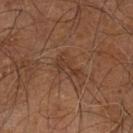Part of a total-body skin-imaging series; this lesion was reviewed on a skin check and was not flagged for biopsy.
From the right leg.
Approximately 3.5 mm at its widest.
This image is a 15 mm lesion crop taken from a total-body photograph.
The patient is a male aged around 60.
The total-body-photography lesion software estimated border irregularity of about 4.5 on a 0–10 scale, a within-lesion color-variation index near 1/10, and a peripheral color-asymmetry measure near 0.5.